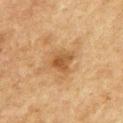Captured during whole-body skin photography for melanoma surveillance; the lesion was not biopsied. Automated image analysis of the tile measured an eccentricity of roughly 0.45 and two-axis asymmetry of about 0.25. This is a cross-polarized tile. A region of skin cropped from a whole-body photographic capture, roughly 15 mm wide. On the front of the torso. A male subject aged around 75. The lesion's longest dimension is about 3 mm.